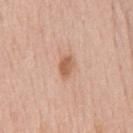Q: Was this lesion biopsied?
A: catalogued during a skin exam; not biopsied
Q: Patient demographics?
A: male, in their 60s
Q: Illumination type?
A: white-light illumination
Q: What is the anatomic site?
A: the chest
Q: Lesion size?
A: ~3 mm (longest diameter)
Q: What is the imaging modality?
A: ~15 mm tile from a whole-body skin photo